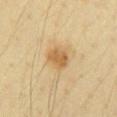Captured during whole-body skin photography for melanoma surveillance; the lesion was not biopsied. Imaged with cross-polarized lighting. The patient is a male aged 43 to 47. The lesion is on the chest. A 15 mm crop from a total-body photograph taken for skin-cancer surveillance. Approximately 3 mm at its widest.